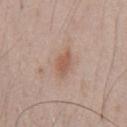The total-body-photography lesion software estimated a lesion area of about 4.5 mm², an eccentricity of roughly 0.8, and a shape-asymmetry score of about 0.3 (0 = symmetric). The analysis additionally found an average lesion color of about L≈56 a*≈20 b*≈28 (CIELAB), a lesion–skin lightness drop of about 9, and a lesion-to-skin contrast of about 7 (normalized; higher = more distinct). The analysis additionally found a border-irregularity index near 3/10, internal color variation of about 1 on a 0–10 scale, and peripheral color asymmetry of about 0.5. The software also gave an automated nevus-likeness rating near 20 out of 100.
The lesion is located on the chest.
A male subject, approximately 50 years of age.
A 15 mm crop from a total-body photograph taken for skin-cancer surveillance.
The recorded lesion diameter is about 3 mm.
Imaged with white-light lighting.